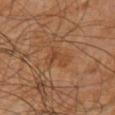  biopsy_status: not biopsied; imaged during a skin examination
  site: arm
  patient:
    sex: male
    age_approx: 60
  image:
    source: total-body photography crop
    field_of_view_mm: 15
  automated_metrics:
    eccentricity: 0.85
    shape_asymmetry: 0.45
    cielab_L: 43
    cielab_a: 22
    cielab_b: 34
    vs_skin_darker_L: 5.0
    vs_skin_contrast_norm: 5.0
    nevus_likeness_0_100: 0
    lesion_detection_confidence_0_100: 85